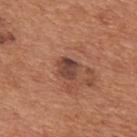<record>
<biopsy_status>not biopsied; imaged during a skin examination</biopsy_status>
<site>back</site>
<lesion_size>
  <long_diameter_mm_approx>3.0</long_diameter_mm_approx>
</lesion_size>
<image>
  <source>total-body photography crop</source>
  <field_of_view_mm>15</field_of_view_mm>
</image>
<patient>
  <sex>male</sex>
  <age_approx>65</age_approx>
</patient>
</record>